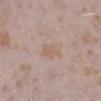Captured during whole-body skin photography for melanoma surveillance; the lesion was not biopsied.
The tile uses white-light illumination.
A female patient, aged approximately 25.
On the leg.
Longest diameter approximately 2.5 mm.
A roughly 15 mm field-of-view crop from a total-body skin photograph.